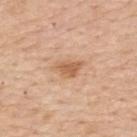follow-up = catalogued during a skin exam; not biopsied | tile lighting = white-light | lesion size = about 3.5 mm | subject = female, approximately 65 years of age | imaging modality = ~15 mm tile from a whole-body skin photo | body site = the back | TBP lesion metrics = an area of roughly 5.5 mm²; an average lesion color of about L≈61 a*≈22 b*≈35 (CIELAB) and a lesion–skin lightness drop of about 10.